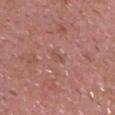biopsy_status: not biopsied; imaged during a skin examination
lighting: white-light
site: head or neck
image:
  source: total-body photography crop
  field_of_view_mm: 15
patient:
  sex: male
  age_approx: 60
lesion_size:
  long_diameter_mm_approx: 2.5